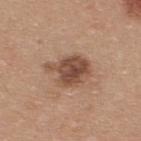This lesion was catalogued during total-body skin photography and was not selected for biopsy. The subject is a male in their mid-30s. This image is a 15 mm lesion crop taken from a total-body photograph. An algorithmic analysis of the crop reported a lesion area of about 13 mm², an outline eccentricity of about 0.65 (0 = round, 1 = elongated), and a symmetry-axis asymmetry near 0.3. It also reported a lesion color around L≈49 a*≈20 b*≈29 in CIELAB, a lesion–skin lightness drop of about 13, and a normalized border contrast of about 9. And it measured border irregularity of about 3.5 on a 0–10 scale and radial color variation of about 2. The analysis additionally found an automated nevus-likeness rating near 30 out of 100 and lesion-presence confidence of about 100/100. From the upper back.